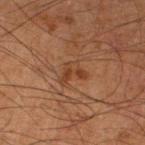Captured during whole-body skin photography for melanoma surveillance; the lesion was not biopsied.
A region of skin cropped from a whole-body photographic capture, roughly 15 mm wide.
The lesion is located on the left lower leg.
Approximately 3 mm at its widest.
Automated tile analysis of the lesion measured an average lesion color of about L≈33 a*≈19 b*≈28 (CIELAB), roughly 6 lightness units darker than nearby skin, and a normalized lesion–skin contrast near 6.
The patient is a male approximately 65 years of age.